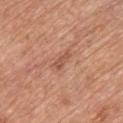The lesion was tiled from a total-body skin photograph and was not biopsied.
Automated image analysis of the tile measured a lesion color around L≈54 a*≈25 b*≈32 in CIELAB, about 9 CIELAB-L* units darker than the surrounding skin, and a lesion-to-skin contrast of about 6 (normalized; higher = more distinct). It also reported a border-irregularity index near 4.5/10, internal color variation of about 0.5 on a 0–10 scale, and a peripheral color-asymmetry measure near 0. And it measured a detector confidence of about 100 out of 100 that the crop contains a lesion.
The tile uses white-light illumination.
A male patient, aged around 60.
The lesion is on the chest.
A 15 mm close-up tile from a total-body photography series done for melanoma screening.
Approximately 2.5 mm at its widest.